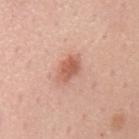Recorded during total-body skin imaging; not selected for excision or biopsy.